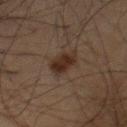Impression:
Captured during whole-body skin photography for melanoma surveillance; the lesion was not biopsied.
Clinical summary:
The lesion is located on the chest. A close-up tile cropped from a whole-body skin photograph, about 15 mm across. A male patient, aged 63 to 67.